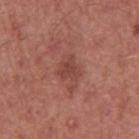Findings:
* notes — total-body-photography surveillance lesion; no biopsy
* site — the left upper arm
* automated lesion analysis — a footprint of about 4 mm² and a symmetry-axis asymmetry near 0.4; a mean CIELAB color near L≈44 a*≈26 b*≈26, roughly 8 lightness units darker than nearby skin, and a normalized lesion–skin contrast near 6; a border-irregularity rating of about 4/10 and a within-lesion color-variation index near 1.5/10
* subject — male, in their mid-40s
* illumination — white-light illumination
* imaging modality — total-body-photography crop, ~15 mm field of view
* diameter — ~2.5 mm (longest diameter)